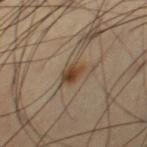<record>
  <biopsy_status>not biopsied; imaged during a skin examination</biopsy_status>
  <site>right thigh</site>
  <patient>
    <sex>male</sex>
    <age_approx>65</age_approx>
  </patient>
  <image>
    <source>total-body photography crop</source>
    <field_of_view_mm>15</field_of_view_mm>
  </image>
</record>